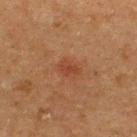Clinical impression: Imaged during a routine full-body skin examination; the lesion was not biopsied and no histopathology is available. Clinical summary: Automated tile analysis of the lesion measured an area of roughly 3.5 mm² and an outline eccentricity of about 0.7 (0 = round, 1 = elongated). The analysis additionally found a mean CIELAB color near L≈35 a*≈22 b*≈28, a lesion–skin lightness drop of about 6, and a lesion-to-skin contrast of about 5.5 (normalized; higher = more distinct). The software also gave a border-irregularity rating of about 2.5/10 and radial color variation of about 0.5. The analysis additionally found an automated nevus-likeness rating near 65 out of 100. Cropped from a whole-body photographic skin survey; the tile spans about 15 mm. Captured under cross-polarized illumination. On the upper back. A male subject aged 48 to 52. The lesion's longest dimension is about 2.5 mm.